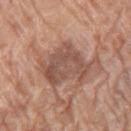workup = imaged on a skin check; not biopsied
body site = the right thigh
subject = female, approximately 75 years of age
image source = ~15 mm tile from a whole-body skin photo